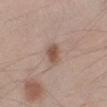<case>
<biopsy_status>not biopsied; imaged during a skin examination</biopsy_status>
<image>
  <source>total-body photography crop</source>
  <field_of_view_mm>15</field_of_view_mm>
</image>
<patient>
  <sex>male</sex>
  <age_approx>45</age_approx>
</patient>
<lighting>white-light</lighting>
<site>right forearm</site>
</case>